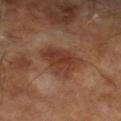Q: Was a biopsy performed?
A: no biopsy performed (imaged during a skin exam)
Q: What kind of image is this?
A: ~15 mm crop, total-body skin-cancer survey
Q: What are the patient's age and sex?
A: male, about 65 years old
Q: What lighting was used for the tile?
A: cross-polarized